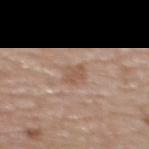workup — catalogued during a skin exam; not biopsied
anatomic site — the upper back
image-analysis metrics — an area of roughly 4.5 mm² and an eccentricity of roughly 0.75; border irregularity of about 2.5 on a 0–10 scale, internal color variation of about 2 on a 0–10 scale, and a peripheral color-asymmetry measure near 0.5; a nevus-likeness score of about 0/100
subject — female, aged approximately 60
image — 15 mm crop, total-body photography
illumination — white-light
lesion size — about 3 mm